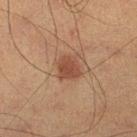Imaged during a routine full-body skin examination; the lesion was not biopsied and no histopathology is available.